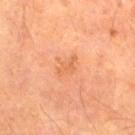biopsy status — imaged on a skin check; not biopsied
location — the right thigh
automated metrics — a lesion area of about 4 mm², an outline eccentricity of about 0.85 (0 = round, 1 = elongated), and a shape-asymmetry score of about 0.4 (0 = symmetric)
subject — male, approximately 65 years of age
illumination — cross-polarized illumination
acquisition — total-body-photography crop, ~15 mm field of view
size — about 3 mm